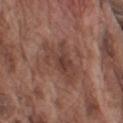The lesion was tiled from a total-body skin photograph and was not biopsied. Located on the left upper arm. The recorded lesion diameter is about 6 mm. This image is a 15 mm lesion crop taken from a total-body photograph. The tile uses white-light illumination. A male subject in their mid-70s.